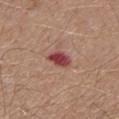Part of a total-body skin-imaging series; this lesion was reviewed on a skin check and was not flagged for biopsy. Longest diameter approximately 3 mm. Cropped from a total-body skin-imaging series; the visible field is about 15 mm. From the left lower leg. An algorithmic analysis of the crop reported a border-irregularity index near 2.5/10, a color-variation rating of about 2.5/10, and peripheral color asymmetry of about 1. It also reported an automated nevus-likeness rating near 0 out of 100 and a detector confidence of about 100 out of 100 that the crop contains a lesion. Captured under white-light illumination. A male subject, approximately 65 years of age.